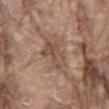workup: no biopsy performed (imaged during a skin exam); patient: male, about 80 years old; acquisition: 15 mm crop, total-body photography; location: the chest.